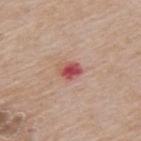Notes:
- imaging modality · ~15 mm tile from a whole-body skin photo
- subject · male, roughly 65 years of age
- location · the upper back